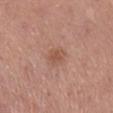{"biopsy_status": "not biopsied; imaged during a skin examination", "site": "right lower leg", "lesion_size": {"long_diameter_mm_approx": 3.0}, "image": {"source": "total-body photography crop", "field_of_view_mm": 15}, "lighting": "white-light", "automated_metrics": {"area_mm2_approx": 4.0, "eccentricity": 0.75, "shape_asymmetry": 0.25, "cielab_L": 53, "cielab_a": 22, "cielab_b": 29, "vs_skin_darker_L": 8.0, "vs_skin_contrast_norm": 6.0, "border_irregularity_0_10": 2.5, "color_variation_0_10": 1.0, "peripheral_color_asymmetry": 0.5, "nevus_likeness_0_100": 10, "lesion_detection_confidence_0_100": 100}, "patient": {"sex": "female", "age_approx": 60}}